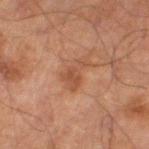follow-up — imaged on a skin check; not biopsied
subject — male, aged 68–72
illumination — cross-polarized illumination
lesion size — about 4 mm
image source — 15 mm crop, total-body photography
body site — the left thigh